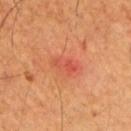Findings:
– workup · total-body-photography surveillance lesion; no biopsy
– subject · male, approximately 70 years of age
– image source · 15 mm crop, total-body photography
– illumination · cross-polarized illumination
– anatomic site · the back